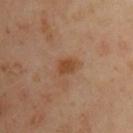Captured during whole-body skin photography for melanoma surveillance; the lesion was not biopsied. From the front of the torso. A close-up tile cropped from a whole-body skin photograph, about 15 mm across. Captured under cross-polarized illumination. About 2.5 mm across. A male subject aged around 45.